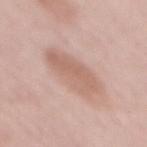This lesion was catalogued during total-body skin photography and was not selected for biopsy. Located on the mid back. Approximately 6.5 mm at its widest. A female patient, in their 60s. A 15 mm crop from a total-body photograph taken for skin-cancer surveillance. Automated image analysis of the tile measured a lesion area of about 17 mm², an outline eccentricity of about 0.9 (0 = round, 1 = elongated), and two-axis asymmetry of about 0.15. And it measured radial color variation of about 1. The analysis additionally found a classifier nevus-likeness of about 70/100 and a detector confidence of about 100 out of 100 that the crop contains a lesion.